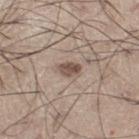{
  "biopsy_status": "not biopsied; imaged during a skin examination",
  "image": {
    "source": "total-body photography crop",
    "field_of_view_mm": 15
  },
  "lighting": "white-light",
  "automated_metrics": {
    "area_mm2_approx": 4.0,
    "shape_asymmetry": 0.2
  },
  "site": "left thigh",
  "patient": {
    "sex": "male",
    "age_approx": 60
  }
}